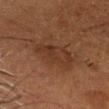{"biopsy_status": "not biopsied; imaged during a skin examination", "lesion_size": {"long_diameter_mm_approx": 4.5}, "lighting": "cross-polarized", "site": "head or neck", "patient": {"sex": "male", "age_approx": 75}, "automated_metrics": {"vs_skin_darker_L": 5.0, "vs_skin_contrast_norm": 5.5}, "image": {"source": "total-body photography crop", "field_of_view_mm": 15}}